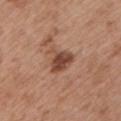Q: Is there a histopathology result?
A: imaged on a skin check; not biopsied
Q: Automated lesion metrics?
A: an eccentricity of roughly 0.4 and a symmetry-axis asymmetry near 0.45; about 12 CIELAB-L* units darker than the surrounding skin and a normalized border contrast of about 9; a classifier nevus-likeness of about 30/100 and lesion-presence confidence of about 100/100
Q: What kind of image is this?
A: 15 mm crop, total-body photography
Q: Illumination type?
A: white-light
Q: What is the lesion's diameter?
A: ~3.5 mm (longest diameter)
Q: Where on the body is the lesion?
A: the left upper arm
Q: Who is the patient?
A: male, in their mid- to late 50s